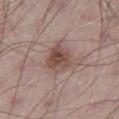Q: How was the tile lit?
A: white-light
Q: Automated lesion metrics?
A: an area of roughly 10 mm²; a mean CIELAB color near L≈49 a*≈18 b*≈23, roughly 11 lightness units darker than nearby skin, and a normalized lesion–skin contrast near 8; border irregularity of about 2.5 on a 0–10 scale, a color-variation rating of about 5.5/10, and radial color variation of about 2
Q: What is the lesion's diameter?
A: ≈5 mm
Q: Patient demographics?
A: male, aged 63 to 67
Q: How was this image acquired?
A: total-body-photography crop, ~15 mm field of view
Q: Lesion location?
A: the left thigh